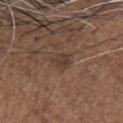Case summary:
- TBP lesion metrics · a lesion area of about 4 mm², an eccentricity of roughly 0.65, and a symmetry-axis asymmetry near 0.35; a lesion color around L≈38 a*≈16 b*≈23 in CIELAB and about 7 CIELAB-L* units darker than the surrounding skin; a border-irregularity rating of about 3/10, internal color variation of about 1.5 on a 0–10 scale, and peripheral color asymmetry of about 0.5
- illumination · white-light
- site · the head or neck
- subject · male, aged 63–67
- acquisition · total-body-photography crop, ~15 mm field of view
- lesion size · ~2.5 mm (longest diameter)